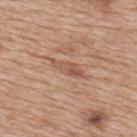Case summary:
* biopsy status · imaged on a skin check; not biopsied
* body site · the upper back
* image source · 15 mm crop, total-body photography
* lesion diameter · ~4 mm (longest diameter)
* subject · female, about 40 years old
* illumination · white-light illumination